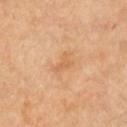{
  "biopsy_status": "not biopsied; imaged during a skin examination",
  "site": "arm",
  "image": {
    "source": "total-body photography crop",
    "field_of_view_mm": 15
  },
  "patient": {
    "sex": "female",
    "age_approx": 65
  }
}